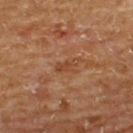| feature | finding |
|---|---|
| follow-up | imaged on a skin check; not biopsied |
| subject | male, in their mid-60s |
| body site | the upper back |
| imaging modality | total-body-photography crop, ~15 mm field of view |
| size | ~3 mm (longest diameter) |
| illumination | cross-polarized illumination |